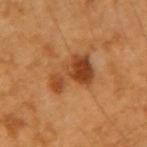Impression:
Recorded during total-body skin imaging; not selected for excision or biopsy.